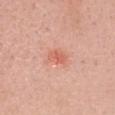Part of a total-body skin-imaging series; this lesion was reviewed on a skin check and was not flagged for biopsy.
This image is a 15 mm lesion crop taken from a total-body photograph.
On the head or neck.
A female subject, aged approximately 25.